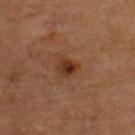Assessment:
Part of a total-body skin-imaging series; this lesion was reviewed on a skin check and was not flagged for biopsy.
Clinical summary:
Located on the upper back. Measured at roughly 2.5 mm in maximum diameter. The subject is aged around 65. This image is a 15 mm lesion crop taken from a total-body photograph.